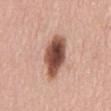{"lighting": "white-light", "patient": {"sex": "female", "age_approx": 65}, "automated_metrics": {"border_irregularity_0_10": 2.0, "color_variation_0_10": 7.5, "peripheral_color_asymmetry": 2.0, "nevus_likeness_0_100": 95, "lesion_detection_confidence_0_100": 100}, "site": "back", "image": {"source": "total-body photography crop", "field_of_view_mm": 15}, "lesion_size": {"long_diameter_mm_approx": 5.5}}